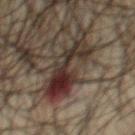The lesion was tiled from a total-body skin photograph and was not biopsied. On the chest. Imaged with cross-polarized lighting. A 15 mm close-up extracted from a 3D total-body photography capture. The subject is a male in their mid-60s.